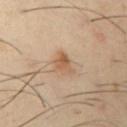Imaged during a routine full-body skin examination; the lesion was not biopsied and no histopathology is available.
The recorded lesion diameter is about 3 mm.
The lesion-visualizer software estimated a footprint of about 4 mm², an eccentricity of roughly 0.65, and two-axis asymmetry of about 0.45. It also reported about 10 CIELAB-L* units darker than the surrounding skin and a normalized border contrast of about 7. It also reported a border-irregularity rating of about 4/10 and radial color variation of about 1. And it measured an automated nevus-likeness rating near 50 out of 100 and lesion-presence confidence of about 100/100.
A 15 mm close-up extracted from a 3D total-body photography capture.
The subject is a male aged 38–42.
The lesion is on the left upper arm.
This is a cross-polarized tile.